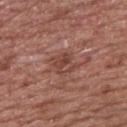Clinical impression: The lesion was tiled from a total-body skin photograph and was not biopsied. Image and clinical context: The recorded lesion diameter is about 3 mm. On the upper back. The tile uses white-light illumination. A close-up tile cropped from a whole-body skin photograph, about 15 mm across. A male subject, in their mid-50s.